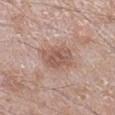follow-up — no biopsy performed (imaged during a skin exam)
imaging modality — 15 mm crop, total-body photography
patient — male, about 60 years old
lesion size — about 4 mm
anatomic site — the right lower leg
tile lighting — white-light illumination
TBP lesion metrics — an area of roughly 11 mm², an outline eccentricity of about 0.7 (0 = round, 1 = elongated), and two-axis asymmetry of about 0.2; an average lesion color of about L≈56 a*≈20 b*≈25 (CIELAB) and a normalized lesion–skin contrast near 6.5; a border-irregularity index near 2.5/10; an automated nevus-likeness rating near 10 out of 100 and lesion-presence confidence of about 100/100